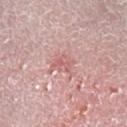Impression: This lesion was catalogued during total-body skin photography and was not selected for biopsy. Image and clinical context: A region of skin cropped from a whole-body photographic capture, roughly 15 mm wide. Imaged with white-light lighting. Automated tile analysis of the lesion measured an average lesion color of about L≈63 a*≈24 b*≈23 (CIELAB) and a lesion–skin lightness drop of about 7. And it measured lesion-presence confidence of about 95/100. The patient is a male aged 48–52. From the left lower leg. Longest diameter approximately 4 mm.